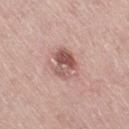Assessment:
Recorded during total-body skin imaging; not selected for excision or biopsy.
Context:
A roughly 15 mm field-of-view crop from a total-body skin photograph. On the right thigh. The subject is a female roughly 65 years of age. Longest diameter approximately 4 mm.